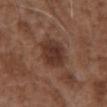{
  "biopsy_status": "not biopsied; imaged during a skin examination",
  "image": {
    "source": "total-body photography crop",
    "field_of_view_mm": 15
  },
  "lighting": "white-light",
  "patient": {
    "sex": "male",
    "age_approx": 75
  },
  "lesion_size": {
    "long_diameter_mm_approx": 4.0
  },
  "site": "abdomen"
}